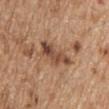Assessment:
Imaged during a routine full-body skin examination; the lesion was not biopsied and no histopathology is available.
Context:
This is a white-light tile. The recorded lesion diameter is about 5 mm. A male patient, in their 70s. From the upper back. This image is a 15 mm lesion crop taken from a total-body photograph.